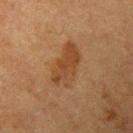Recorded during total-body skin imaging; not selected for excision or biopsy. The subject is a female about 55 years old. The tile uses cross-polarized illumination. The lesion is located on the arm. Cropped from a total-body skin-imaging series; the visible field is about 15 mm. Automated image analysis of the tile measured a lesion area of about 13 mm², a shape eccentricity near 0.85, and a symmetry-axis asymmetry near 0.2. And it measured a border-irregularity rating of about 2.5/10 and a color-variation rating of about 3/10. And it measured a classifier nevus-likeness of about 50/100. Longest diameter approximately 5.5 mm.